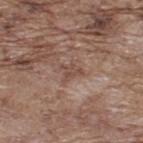Impression:
Part of a total-body skin-imaging series; this lesion was reviewed on a skin check and was not flagged for biopsy.
Image and clinical context:
The patient is a male in their 70s. From the upper back. The total-body-photography lesion software estimated an area of roughly 3.5 mm², a shape eccentricity near 0.7, and a symmetry-axis asymmetry near 0.45. And it measured border irregularity of about 5 on a 0–10 scale, a color-variation rating of about 1.5/10, and peripheral color asymmetry of about 0.5. This image is a 15 mm lesion crop taken from a total-body photograph. The lesion's longest dimension is about 2.5 mm. This is a white-light tile.